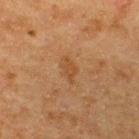The total-body-photography lesion software estimated an area of roughly 4.5 mm², an outline eccentricity of about 0.8 (0 = round, 1 = elongated), and two-axis asymmetry of about 0.25. It also reported border irregularity of about 2.5 on a 0–10 scale, internal color variation of about 1 on a 0–10 scale, and peripheral color asymmetry of about 0.5.
The lesion is located on the upper back.
Cropped from a total-body skin-imaging series; the visible field is about 15 mm.
Captured under cross-polarized illumination.
A male patient, aged around 50.